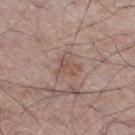workup: catalogued during a skin exam; not biopsied | tile lighting: white-light | anatomic site: the right thigh | image: 15 mm crop, total-body photography | automated lesion analysis: a footprint of about 4 mm², an outline eccentricity of about 0.7 (0 = round, 1 = elongated), and a symmetry-axis asymmetry near 0.45; an average lesion color of about L≈52 a*≈18 b*≈24 (CIELAB), a lesion–skin lightness drop of about 7, and a lesion-to-skin contrast of about 5.5 (normalized; higher = more distinct); a border-irregularity index near 4.5/10, internal color variation of about 1 on a 0–10 scale, and radial color variation of about 0.5 | patient: male, roughly 55 years of age.